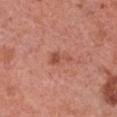  biopsy_status: not biopsied; imaged during a skin examination
  site: chest
  automated_metrics:
    area_mm2_approx: 3.0
    eccentricity: 0.85
    shape_asymmetry: 0.6
    border_irregularity_0_10: 5.5
  lighting: white-light
  lesion_size:
    long_diameter_mm_approx: 2.5
  image:
    source: total-body photography crop
    field_of_view_mm: 15
  patient:
    sex: female
    age_approx: 60